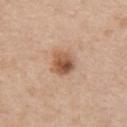Findings:
- biopsy status · total-body-photography surveillance lesion; no biopsy